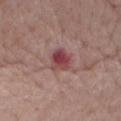Imaged during a routine full-body skin examination; the lesion was not biopsied and no histopathology is available.
This image is a 15 mm lesion crop taken from a total-body photograph.
The lesion is on the mid back.
Automated tile analysis of the lesion measured a lesion color around L≈44 a*≈26 b*≈19 in CIELAB and a normalized lesion–skin contrast near 9. And it measured a peripheral color-asymmetry measure near 1.5.
A female subject in their 70s.
The tile uses white-light illumination.
The recorded lesion diameter is about 3 mm.